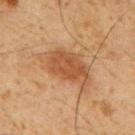Clinical summary: A male patient, aged around 60. This image is a 15 mm lesion crop taken from a total-body photograph. The lesion is located on the back.